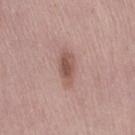Part of a total-body skin-imaging series; this lesion was reviewed on a skin check and was not flagged for biopsy.
This is a white-light tile.
A close-up tile cropped from a whole-body skin photograph, about 15 mm across.
The subject is a female aged 48 to 52.
From the right thigh.
Measured at roughly 4 mm in maximum diameter.